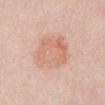* workup — no biopsy performed (imaged during a skin exam)
* body site — the chest
* illumination — white-light illumination
* image — total-body-photography crop, ~15 mm field of view
* lesion size — about 6 mm
* patient — female, about 50 years old